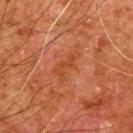biopsy status: total-body-photography surveillance lesion; no biopsy | lighting: cross-polarized illumination | automated lesion analysis: a mean CIELAB color near L≈36 a*≈25 b*≈32, about 5 CIELAB-L* units darker than the surrounding skin, and a lesion-to-skin contrast of about 5.5 (normalized; higher = more distinct); a border-irregularity index near 6/10 and a peripheral color-asymmetry measure near 0.5 | image source: 15 mm crop, total-body photography | diameter: about 3.5 mm | location: the left upper arm | subject: male, in their 80s.